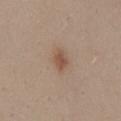biopsy status: imaged on a skin check; not biopsied
size: ~2.5 mm (longest diameter)
image source: ~15 mm crop, total-body skin-cancer survey
patient: female, roughly 25 years of age
lighting: white-light
site: the lower back